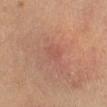biopsy status — total-body-photography surveillance lesion; no biopsy | subject — female, roughly 65 years of age | anatomic site — the leg | lighting — cross-polarized illumination | imaging modality — ~15 mm tile from a whole-body skin photo | lesion size — ~2 mm (longest diameter).